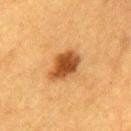A female subject, aged approximately 55.
Measured at roughly 4.5 mm in maximum diameter.
Cropped from a whole-body photographic skin survey; the tile spans about 15 mm.
Captured under cross-polarized illumination.
The lesion is located on the back.
Automated image analysis of the tile measured an area of roughly 10 mm² and an outline eccentricity of about 0.75 (0 = round, 1 = elongated). It also reported border irregularity of about 2.5 on a 0–10 scale, a color-variation rating of about 4/10, and peripheral color asymmetry of about 1.